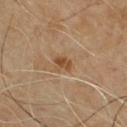Impression: This lesion was catalogued during total-body skin photography and was not selected for biopsy. Background: Located on the chest. Measured at roughly 2.5 mm in maximum diameter. The lesion-visualizer software estimated a shape eccentricity near 0.65. And it measured a mean CIELAB color near L≈46 a*≈18 b*≈33, about 9 CIELAB-L* units darker than the surrounding skin, and a normalized border contrast of about 7.5. The analysis additionally found a within-lesion color-variation index near 3/10. The software also gave a classifier nevus-likeness of about 5/100 and a detector confidence of about 100 out of 100 that the crop contains a lesion. A male subject aged around 60. A 15 mm crop from a total-body photograph taken for skin-cancer surveillance. The tile uses cross-polarized illumination.